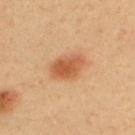Q: Is there a histopathology result?
A: imaged on a skin check; not biopsied
Q: Illumination type?
A: cross-polarized
Q: How was this image acquired?
A: total-body-photography crop, ~15 mm field of view
Q: Patient demographics?
A: male, aged around 40
Q: Where on the body is the lesion?
A: the upper back
Q: What did automated image analysis measure?
A: a lesion color around L≈57 a*≈26 b*≈39 in CIELAB, about 12 CIELAB-L* units darker than the surrounding skin, and a lesion-to-skin contrast of about 8 (normalized; higher = more distinct); border irregularity of about 1.5 on a 0–10 scale, a color-variation rating of about 3.5/10, and a peripheral color-asymmetry measure near 1; lesion-presence confidence of about 100/100
Q: How large is the lesion?
A: ~4 mm (longest diameter)